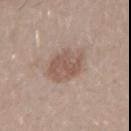Findings:
– follow-up: imaged on a skin check; not biopsied
– patient: male, aged 28–32
– anatomic site: the right upper arm
– imaging modality: total-body-photography crop, ~15 mm field of view
– diameter: about 5 mm
– automated metrics: an eccentricity of roughly 0.6 and two-axis asymmetry of about 0.15